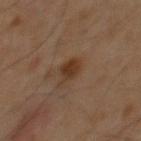workup: no biopsy performed (imaged during a skin exam)